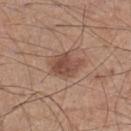The lesion was tiled from a total-body skin photograph and was not biopsied. A close-up tile cropped from a whole-body skin photograph, about 15 mm across. The lesion is located on the leg. Captured under white-light illumination. A male patient approximately 60 years of age. The recorded lesion diameter is about 4 mm. The lesion-visualizer software estimated a mean CIELAB color near L≈48 a*≈20 b*≈26, roughly 11 lightness units darker than nearby skin, and a normalized border contrast of about 8. And it measured a nevus-likeness score of about 55/100.